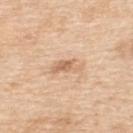| feature | finding |
|---|---|
| notes | total-body-photography surveillance lesion; no biopsy |
| image source | ~15 mm crop, total-body skin-cancer survey |
| TBP lesion metrics | an outline eccentricity of about 0.9 (0 = round, 1 = elongated) and two-axis asymmetry of about 0.4; a mean CIELAB color near L≈66 a*≈19 b*≈35, a lesion–skin lightness drop of about 10, and a normalized border contrast of about 6; a classifier nevus-likeness of about 0/100 and lesion-presence confidence of about 100/100 |
| anatomic site | the back |
| size | ~4 mm (longest diameter) |
| subject | female, aged around 55 |